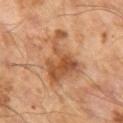Imaged during a routine full-body skin examination; the lesion was not biopsied and no histopathology is available. Automated image analysis of the tile measured border irregularity of about 8 on a 0–10 scale and peripheral color asymmetry of about 2. And it measured a nevus-likeness score of about 10/100 and a detector confidence of about 100 out of 100 that the crop contains a lesion. Cropped from a whole-body photographic skin survey; the tile spans about 15 mm. Captured under cross-polarized illumination. The subject is a male aged 63 to 67. Longest diameter approximately 6.5 mm.